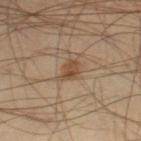Impression: Part of a total-body skin-imaging series; this lesion was reviewed on a skin check and was not flagged for biopsy. Clinical summary: Automated tile analysis of the lesion measured a lesion area of about 4 mm² and a shape eccentricity near 0.8. The analysis additionally found border irregularity of about 3 on a 0–10 scale, a within-lesion color-variation index near 3/10, and peripheral color asymmetry of about 1. The lesion's longest dimension is about 3 mm. A male subject, about 40 years old. Imaged with cross-polarized lighting. From the left thigh. A 15 mm close-up tile from a total-body photography series done for melanoma screening.